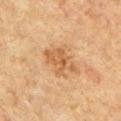biopsy_status: not biopsied; imaged during a skin examination
site: chest
image:
  source: total-body photography crop
  field_of_view_mm: 15
patient:
  sex: male
  age_approx: 65
lesion_size:
  long_diameter_mm_approx: 4.5
lighting: cross-polarized